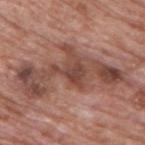Q: Is there a histopathology result?
A: no biopsy performed (imaged during a skin exam)
Q: Lesion location?
A: the back
Q: How was this image acquired?
A: 15 mm crop, total-body photography
Q: Who is the patient?
A: male, in their 70s